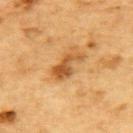workup: total-body-photography surveillance lesion; no biopsy
anatomic site: the upper back
automated metrics: a lesion color around L≈48 a*≈21 b*≈40 in CIELAB, roughly 11 lightness units darker than nearby skin, and a lesion-to-skin contrast of about 8 (normalized; higher = more distinct); a border-irregularity index near 5/10 and a within-lesion color-variation index near 3/10
tile lighting: cross-polarized illumination
lesion diameter: ≈4.5 mm
patient: male, in their mid-80s or older
acquisition: 15 mm crop, total-body photography